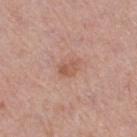notes: total-body-photography surveillance lesion; no biopsy
subject: female, aged around 40
location: the leg
automated lesion analysis: an outline eccentricity of about 0.75 (0 = round, 1 = elongated) and a shape-asymmetry score of about 0.35 (0 = symmetric); internal color variation of about 3.5 on a 0–10 scale and a peripheral color-asymmetry measure near 1.5; a classifier nevus-likeness of about 10/100 and a detector confidence of about 100 out of 100 that the crop contains a lesion
acquisition: ~15 mm tile from a whole-body skin photo